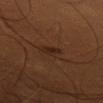This lesion was catalogued during total-body skin photography and was not selected for biopsy.
The tile uses cross-polarized illumination.
On the left thigh.
A close-up tile cropped from a whole-body skin photograph, about 15 mm across.
A male subject aged around 60.